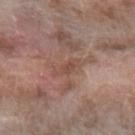| field | value |
|---|---|
| notes | imaged on a skin check; not biopsied |
| body site | the arm |
| lighting | white-light illumination |
| automated metrics | a lesion area of about 3.5 mm², an outline eccentricity of about 0.9 (0 = round, 1 = elongated), and a shape-asymmetry score of about 0.3 (0 = symmetric); a nevus-likeness score of about 0/100 and a detector confidence of about 75 out of 100 that the crop contains a lesion |
| patient | female, approximately 75 years of age |
| lesion diameter | ~3 mm (longest diameter) |
| acquisition | 15 mm crop, total-body photography |